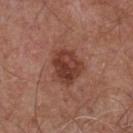Q: Was this lesion biopsied?
A: total-body-photography surveillance lesion; no biopsy
Q: What is the anatomic site?
A: the chest
Q: What is the imaging modality?
A: 15 mm crop, total-body photography
Q: Patient demographics?
A: male, aged around 55